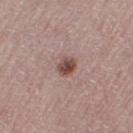Imaged during a routine full-body skin examination; the lesion was not biopsied and no histopathology is available. Cropped from a whole-body photographic skin survey; the tile spans about 15 mm. Approximately 2.5 mm at its widest. On the right thigh. Imaged with white-light lighting. Automated tile analysis of the lesion measured a normalized lesion–skin contrast near 9.5. A female subject, in their mid- to late 50s.